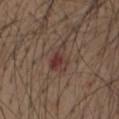biopsy status: catalogued during a skin exam; not biopsied | anatomic site: the abdomen | subject: male, aged around 75 | image: ~15 mm tile from a whole-body skin photo.